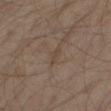notes: imaged on a skin check; not biopsied